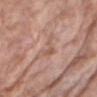size: ≈3 mm
body site: the chest
image: ~15 mm tile from a whole-body skin photo
patient: male, about 75 years old
automated metrics: a footprint of about 4 mm²; a mean CIELAB color near L≈57 a*≈20 b*≈27, a lesion–skin lightness drop of about 7, and a lesion-to-skin contrast of about 5 (normalized; higher = more distinct); a border-irregularity rating of about 8/10, a color-variation rating of about 1/10, and a peripheral color-asymmetry measure near 0.5; a classifier nevus-likeness of about 0/100 and lesion-presence confidence of about 100/100